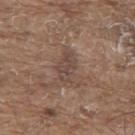Clinical impression: Captured during whole-body skin photography for melanoma surveillance; the lesion was not biopsied. Acquisition and patient details: The lesion is on the upper back. The recorded lesion diameter is about 4.5 mm. The lesion-visualizer software estimated a border-irregularity index near 5/10, a color-variation rating of about 2.5/10, and peripheral color asymmetry of about 1. The analysis additionally found a nevus-likeness score of about 0/100 and a detector confidence of about 85 out of 100 that the crop contains a lesion. A male patient, aged around 80. Imaged with white-light lighting. A region of skin cropped from a whole-body photographic capture, roughly 15 mm wide.